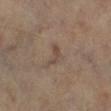Findings:
- notes — total-body-photography surveillance lesion; no biopsy
- location — the right lower leg
- image source — ~15 mm tile from a whole-body skin photo
- lighting — cross-polarized illumination
- subject — male, aged 63–67
- size — ~3 mm (longest diameter)